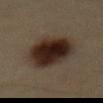lesion diameter = ≈7.5 mm
illumination = cross-polarized illumination
patient = male, aged 38 to 42
acquisition = 15 mm crop, total-body photography
location = the abdomen